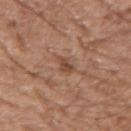notes: imaged on a skin check; not biopsied
lighting: white-light illumination
body site: the right upper arm
image-analysis metrics: a mean CIELAB color near L≈46 a*≈22 b*≈29, roughly 9 lightness units darker than nearby skin, and a normalized border contrast of about 7; a color-variation rating of about 0.5/10 and radial color variation of about 0
acquisition: 15 mm crop, total-body photography
subject: male, aged approximately 75